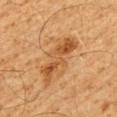Case summary:
- follow-up — imaged on a skin check; not biopsied
- lighting — cross-polarized illumination
- lesion size — about 6 mm
- patient — male, about 60 years old
- automated lesion analysis — a footprint of about 11 mm² and an outline eccentricity of about 0.95 (0 = round, 1 = elongated); an average lesion color of about L≈44 a*≈21 b*≈36 (CIELAB), about 9 CIELAB-L* units darker than the surrounding skin, and a normalized lesion–skin contrast near 7.5
- imaging modality — 15 mm crop, total-body photography
- body site — the mid back